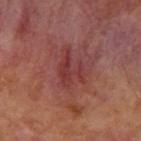biopsy status — catalogued during a skin exam; not biopsied